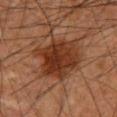{"biopsy_status": "not biopsied; imaged during a skin examination", "site": "mid back", "lesion_size": {"long_diameter_mm_approx": 6.5}, "patient": {"sex": "male", "age_approx": 60}, "image": {"source": "total-body photography crop", "field_of_view_mm": 15}}